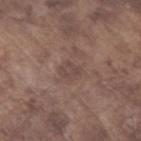Captured during whole-body skin photography for melanoma surveillance; the lesion was not biopsied. Automated image analysis of the tile measured a footprint of about 3.5 mm², a shape eccentricity near 0.7, and a shape-asymmetry score of about 0.25 (0 = symmetric). And it measured a lesion color around L≈44 a*≈16 b*≈20 in CIELAB and a normalized border contrast of about 5.5. It also reported a classifier nevus-likeness of about 0/100 and a lesion-detection confidence of about 100/100. The tile uses white-light illumination. A male patient, aged around 75. A region of skin cropped from a whole-body photographic capture, roughly 15 mm wide. On the left forearm.